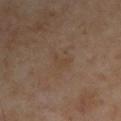follow-up = total-body-photography surveillance lesion; no biopsy
location = the right upper arm
patient = male, in their mid- to late 50s
imaging modality = 15 mm crop, total-body photography
TBP lesion metrics = a footprint of about 3 mm² and a symmetry-axis asymmetry near 0.6; a mean CIELAB color near L≈41 a*≈15 b*≈28, about 4 CIELAB-L* units darker than the surrounding skin, and a normalized border contrast of about 4.5
lighting = cross-polarized